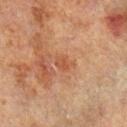The lesion was tiled from a total-body skin photograph and was not biopsied.
A 15 mm close-up extracted from a 3D total-body photography capture.
The subject is a male roughly 70 years of age.
Located on the right lower leg.